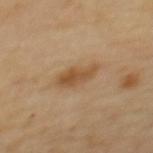Part of a total-body skin-imaging series; this lesion was reviewed on a skin check and was not flagged for biopsy. Located on the upper back. A female patient in their 60s. A lesion tile, about 15 mm wide, cut from a 3D total-body photograph. About 4.5 mm across.